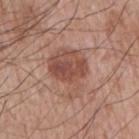Clinical impression:
No biopsy was performed on this lesion — it was imaged during a full skin examination and was not determined to be concerning.
Clinical summary:
A male subject in their mid-60s. Approximately 6 mm at its widest. Cropped from a whole-body photographic skin survey; the tile spans about 15 mm. From the right upper arm. Captured under white-light illumination. Automated image analysis of the tile measured a border-irregularity rating of about 4.5/10.